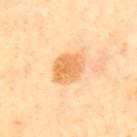Q: Is there a histopathology result?
A: no biopsy performed (imaged during a skin exam)
Q: Patient demographics?
A: male, aged around 60
Q: Where on the body is the lesion?
A: the mid back
Q: What did automated image analysis measure?
A: a mean CIELAB color near L≈70 a*≈23 b*≈46 and a lesion–skin lightness drop of about 11; a border-irregularity index near 1.5/10 and peripheral color asymmetry of about 1.5
Q: What kind of image is this?
A: 15 mm crop, total-body photography
Q: How was the tile lit?
A: cross-polarized illumination